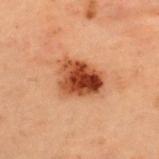Captured during whole-body skin photography for melanoma surveillance; the lesion was not biopsied. A female patient, aged 38 to 42. Measured at roughly 5 mm in maximum diameter. The lesion is located on the upper back. A 15 mm close-up extracted from a 3D total-body photography capture. Automated tile analysis of the lesion measured a normalized border contrast of about 11.5. It also reported a color-variation rating of about 9.5/10. The software also gave lesion-presence confidence of about 100/100. This is a cross-polarized tile.